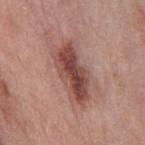Q: Was a biopsy performed?
A: catalogued during a skin exam; not biopsied
Q: How was the tile lit?
A: white-light illumination
Q: Patient demographics?
A: male, aged around 55
Q: Lesion size?
A: ~7 mm (longest diameter)
Q: Where on the body is the lesion?
A: the chest
Q: How was this image acquired?
A: ~15 mm tile from a whole-body skin photo
Q: What did automated image analysis measure?
A: a mean CIELAB color near L≈47 a*≈23 b*≈24